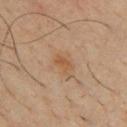| key | value |
|---|---|
| biopsy status | catalogued during a skin exam; not biopsied |
| location | the chest |
| size | ~2.5 mm (longest diameter) |
| TBP lesion metrics | a mean CIELAB color near L≈48 a*≈18 b*≈33, about 6 CIELAB-L* units darker than the surrounding skin, and a normalized border contrast of about 6.5; an automated nevus-likeness rating near 40 out of 100 and a detector confidence of about 100 out of 100 that the crop contains a lesion |
| image source | ~15 mm crop, total-body skin-cancer survey |
| subject | male, aged around 35 |
| illumination | cross-polarized |